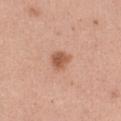Clinical impression:
Part of a total-body skin-imaging series; this lesion was reviewed on a skin check and was not flagged for biopsy.
Acquisition and patient details:
A 15 mm close-up tile from a total-body photography series done for melanoma screening. Measured at roughly 2.5 mm in maximum diameter. The lesion is on the left thigh. A female subject aged 28 to 32.